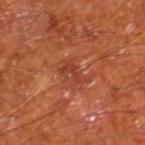notes = total-body-photography surveillance lesion; no biopsy | image source = 15 mm crop, total-body photography | lesion size = ≈3 mm | image-analysis metrics = a lesion area of about 4 mm², an eccentricity of roughly 0.8, and two-axis asymmetry of about 0.55; a mean CIELAB color near L≈39 a*≈30 b*≈33, a lesion–skin lightness drop of about 7, and a normalized border contrast of about 6; a border-irregularity rating of about 7/10, a color-variation rating of about 0/10, and radial color variation of about 0; an automated nevus-likeness rating near 0 out of 100 and a detector confidence of about 100 out of 100 that the crop contains a lesion | patient = male, roughly 65 years of age | site = the right lower leg | tile lighting = cross-polarized illumination.